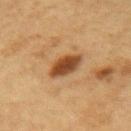| field | value |
|---|---|
| biopsy status | catalogued during a skin exam; not biopsied |
| anatomic site | the right forearm |
| lesion size | about 3.5 mm |
| lighting | cross-polarized |
| image | ~15 mm tile from a whole-body skin photo |
| subject | female, in their mid-40s |